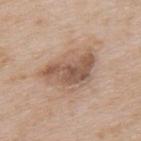<lesion>
<biopsy_status>not biopsied; imaged during a skin examination</biopsy_status>
<site>upper back</site>
<lighting>white-light</lighting>
<automated_metrics>
  <border_irregularity_0_10>4.0</border_irregularity_0_10>
  <peripheral_color_asymmetry>2.0</peripheral_color_asymmetry>
</automated_metrics>
<lesion_size>
  <long_diameter_mm_approx>6.0</long_diameter_mm_approx>
</lesion_size>
<patient>
  <sex>male</sex>
  <age_approx>65</age_approx>
</patient>
<image>
  <source>total-body photography crop</source>
  <field_of_view_mm>15</field_of_view_mm>
</image>
</lesion>